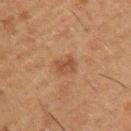Q: Was a biopsy performed?
A: no biopsy performed (imaged during a skin exam)
Q: What is the anatomic site?
A: the left upper arm
Q: How was this image acquired?
A: ~15 mm crop, total-body skin-cancer survey
Q: Patient demographics?
A: male, in their 50s